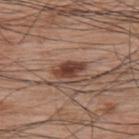  biopsy_status: not biopsied; imaged during a skin examination
  lesion_size:
    long_diameter_mm_approx: 4.0
  patient:
    sex: male
    age_approx: 70
  automated_metrics:
    vs_skin_contrast_norm: 10.5
    border_irregularity_0_10: 3.0
    color_variation_0_10: 3.0
    peripheral_color_asymmetry: 1.0
    nevus_likeness_0_100: 95
    lesion_detection_confidence_0_100: 100
  image:
    source: total-body photography crop
    field_of_view_mm: 15
  site: upper back